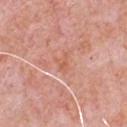Impression:
The lesion was photographed on a routine skin check and not biopsied; there is no pathology result.
Background:
Approximately 2.5 mm at its widest. Captured under white-light illumination. A 15 mm close-up tile from a total-body photography series done for melanoma screening. The lesion is located on the chest. The subject is a male in their 80s.